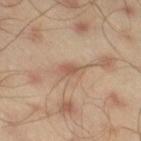follow-up: imaged on a skin check; not biopsied | size: about 3 mm | subject: male, in their mid-40s | tile lighting: cross-polarized illumination | body site: the right thigh | acquisition: ~15 mm tile from a whole-body skin photo | automated metrics: an outline eccentricity of about 0.85 (0 = round, 1 = elongated); a lesion color around L≈55 a*≈19 b*≈29 in CIELAB and about 8 CIELAB-L* units darker than the surrounding skin; lesion-presence confidence of about 100/100.